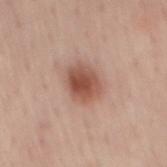Notes:
– lesion size — ≈3.5 mm
– acquisition — 15 mm crop, total-body photography
– illumination — white-light
– body site — the back
– TBP lesion metrics — a border-irregularity index near 1.5/10, internal color variation of about 4.5 on a 0–10 scale, and a peripheral color-asymmetry measure near 1.5; an automated nevus-likeness rating near 100 out of 100 and a detector confidence of about 100 out of 100 that the crop contains a lesion
– patient — male, in their mid- to late 50s